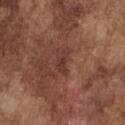Clinical impression:
No biopsy was performed on this lesion — it was imaged during a full skin examination and was not determined to be concerning.
Clinical summary:
The total-body-photography lesion software estimated a mean CIELAB color near L≈36 a*≈23 b*≈24, a lesion–skin lightness drop of about 7, and a normalized lesion–skin contrast near 6. The analysis additionally found a border-irregularity rating of about 5.5/10, internal color variation of about 1.5 on a 0–10 scale, and a peripheral color-asymmetry measure near 0.5. Imaged with white-light lighting. A male subject, roughly 75 years of age. A roughly 15 mm field-of-view crop from a total-body skin photograph. Measured at roughly 3 mm in maximum diameter. The lesion is on the chest.